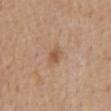Recorded during total-body skin imaging; not selected for excision or biopsy. This is a white-light tile. Measured at roughly 2.5 mm in maximum diameter. The patient is a male aged 63–67. Cropped from a total-body skin-imaging series; the visible field is about 15 mm. Automated image analysis of the tile measured a lesion-detection confidence of about 100/100. The lesion is located on the mid back.